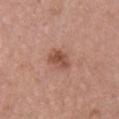Case summary:
* image source: ~15 mm tile from a whole-body skin photo
* subject: female, aged 43 to 47
* illumination: white-light illumination
* size: about 3 mm
* location: the chest